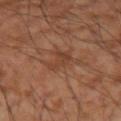No biopsy was performed on this lesion — it was imaged during a full skin examination and was not determined to be concerning. This is a cross-polarized tile. An algorithmic analysis of the crop reported a lesion color around L≈41 a*≈22 b*≈30 in CIELAB and about 6 CIELAB-L* units darker than the surrounding skin. And it measured a lesion-detection confidence of about 100/100. The lesion is located on the right forearm. Cropped from a total-body skin-imaging series; the visible field is about 15 mm. A male patient in their mid- to late 50s.